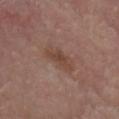Captured during whole-body skin photography for melanoma surveillance; the lesion was not biopsied. This image is a 15 mm lesion crop taken from a total-body photograph. On the head or neck. The total-body-photography lesion software estimated a lesion area of about 4.5 mm². It also reported a border-irregularity index near 5/10, a color-variation rating of about 2/10, and peripheral color asymmetry of about 0.5. The software also gave a nevus-likeness score of about 0/100 and a detector confidence of about 100 out of 100 that the crop contains a lesion. A male subject roughly 80 years of age. This is a white-light tile.